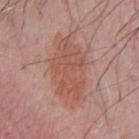| key | value |
|---|---|
| notes | imaged on a skin check; not biopsied |
| anatomic site | the chest |
| image source | ~15 mm crop, total-body skin-cancer survey |
| subject | male, approximately 80 years of age |